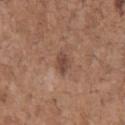The lesion was photographed on a routine skin check and not biopsied; there is no pathology result. The tile uses white-light illumination. A male subject, about 70 years old. The lesion's longest dimension is about 3 mm. On the chest. This image is a 15 mm lesion crop taken from a total-body photograph.